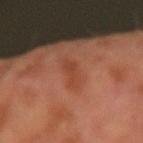Assessment: This lesion was catalogued during total-body skin photography and was not selected for biopsy. Context: The lesion's longest dimension is about 4.5 mm. A male patient about 65 years old. The tile uses cross-polarized illumination. Automated image analysis of the tile measured an area of roughly 5 mm² and an eccentricity of roughly 0.95. The software also gave a border-irregularity rating of about 4.5/10, a color-variation rating of about 1.5/10, and a peripheral color-asymmetry measure near 0.5. The analysis additionally found a nevus-likeness score of about 0/100 and lesion-presence confidence of about 100/100. A 15 mm crop from a total-body photograph taken for skin-cancer surveillance.